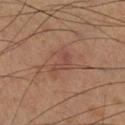This lesion was catalogued during total-body skin photography and was not selected for biopsy.